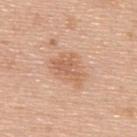Clinical impression: Imaged during a routine full-body skin examination; the lesion was not biopsied and no histopathology is available. Image and clinical context: The subject is a male in their 50s. Automated image analysis of the tile measured a footprint of about 8.5 mm², an outline eccentricity of about 0.75 (0 = round, 1 = elongated), and two-axis asymmetry of about 0.3. The software also gave a lesion color around L≈62 a*≈22 b*≈33 in CIELAB, a lesion–skin lightness drop of about 9, and a normalized lesion–skin contrast near 6. And it measured internal color variation of about 2.5 on a 0–10 scale and peripheral color asymmetry of about 1. It also reported an automated nevus-likeness rating near 15 out of 100 and a lesion-detection confidence of about 100/100. A region of skin cropped from a whole-body photographic capture, roughly 15 mm wide. Approximately 4 mm at its widest. Located on the back.